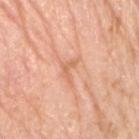Impression:
Imaged during a routine full-body skin examination; the lesion was not biopsied and no histopathology is available.
Clinical summary:
An algorithmic analysis of the crop reported an average lesion color of about L≈67 a*≈25 b*≈35 (CIELAB), a lesion–skin lightness drop of about 8, and a lesion-to-skin contrast of about 5 (normalized; higher = more distinct). The lesion's longest dimension is about 3 mm. Cropped from a total-body skin-imaging series; the visible field is about 15 mm. This is a white-light tile. From the right upper arm. The patient is a female approximately 70 years of age.